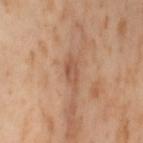Recorded during total-body skin imaging; not selected for excision or biopsy. A region of skin cropped from a whole-body photographic capture, roughly 15 mm wide. The lesion-visualizer software estimated a lesion area of about 3 mm² and a shape-asymmetry score of about 0.2 (0 = symmetric). And it measured a border-irregularity rating of about 2/10 and a within-lesion color-variation index near 2/10. The subject is a female aged approximately 55. The lesion is located on the left thigh.